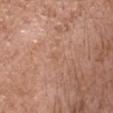Impression:
Captured during whole-body skin photography for melanoma surveillance; the lesion was not biopsied.